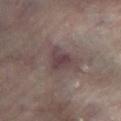Part of a total-body skin-imaging series; this lesion was reviewed on a skin check and was not flagged for biopsy. The lesion is on the left lower leg. A 15 mm close-up extracted from a 3D total-body photography capture. The patient is a male about 65 years old. Automated tile analysis of the lesion measured an area of roughly 7.5 mm², an outline eccentricity of about 0.45 (0 = round, 1 = elongated), and a shape-asymmetry score of about 0.4 (0 = symmetric). The analysis additionally found an automated nevus-likeness rating near 0 out of 100 and a detector confidence of about 90 out of 100 that the crop contains a lesion.